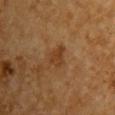lesion size: ~2.5 mm (longest diameter) | subject: male, approximately 85 years of age or older | illumination: cross-polarized illumination | automated metrics: a lesion area of about 4.5 mm², an eccentricity of roughly 0.6, and two-axis asymmetry of about 0.4; a mean CIELAB color near L≈33 a*≈18 b*≈31, about 6 CIELAB-L* units darker than the surrounding skin, and a lesion-to-skin contrast of about 6.5 (normalized; higher = more distinct); a nevus-likeness score of about 0/100 and a detector confidence of about 100 out of 100 that the crop contains a lesion | image source: 15 mm crop, total-body photography | location: the front of the torso.